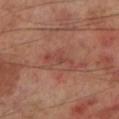{
  "biopsy_status": "not biopsied; imaged during a skin examination",
  "image": {
    "source": "total-body photography crop",
    "field_of_view_mm": 15
  },
  "site": "left lower leg",
  "patient": {
    "sex": "male",
    "age_approx": 70
  },
  "lighting": "cross-polarized",
  "automated_metrics": {
    "area_mm2_approx": 5.5,
    "eccentricity": 0.9,
    "shape_asymmetry": 0.45,
    "nevus_likeness_0_100": 0,
    "lesion_detection_confidence_0_100": 100
  }
}